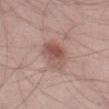Impression:
The lesion was tiled from a total-body skin photograph and was not biopsied.
Context:
This is a white-light tile. About 4.5 mm across. From the left thigh. This image is a 15 mm lesion crop taken from a total-body photograph. A male subject, about 35 years old.